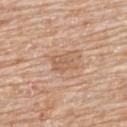Assessment:
This lesion was catalogued during total-body skin photography and was not selected for biopsy.
Clinical summary:
The lesion is on the upper back. A female patient, approximately 85 years of age or older. The tile uses white-light illumination. The lesion's longest dimension is about 3 mm. A region of skin cropped from a whole-body photographic capture, roughly 15 mm wide.